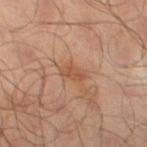No biopsy was performed on this lesion — it was imaged during a full skin examination and was not determined to be concerning.
Cropped from a whole-body photographic skin survey; the tile spans about 15 mm.
Measured at roughly 3 mm in maximum diameter.
The lesion is on the left lower leg.
The patient is a male in their mid-60s.
The tile uses cross-polarized illumination.